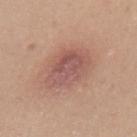Q: Is there a histopathology result?
A: no biopsy performed (imaged during a skin exam)
Q: Where on the body is the lesion?
A: the left upper arm
Q: Illumination type?
A: white-light
Q: Patient demographics?
A: male, in their mid- to late 40s
Q: Automated lesion metrics?
A: a lesion area of about 16 mm², an outline eccentricity of about 0.5 (0 = round, 1 = elongated), and two-axis asymmetry of about 0.15; a lesion color around L≈54 a*≈22 b*≈24 in CIELAB, a lesion–skin lightness drop of about 9, and a lesion-to-skin contrast of about 7 (normalized; higher = more distinct); a classifier nevus-likeness of about 0/100 and lesion-presence confidence of about 100/100
Q: Lesion size?
A: ≈4.5 mm
Q: How was this image acquired?
A: ~15 mm tile from a whole-body skin photo